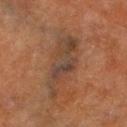Located on the right lower leg. A 15 mm close-up tile from a total-body photography series done for melanoma screening. This is a cross-polarized tile. The subject is a male roughly 70 years of age. The recorded lesion diameter is about 7.5 mm.